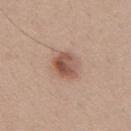Background: The lesion is on the mid back. A male subject aged approximately 40. Cropped from a whole-body photographic skin survey; the tile spans about 15 mm.